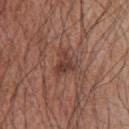Notes:
* TBP lesion metrics · a footprint of about 4.5 mm² and a shape eccentricity near 0.7; a mean CIELAB color near L≈39 a*≈21 b*≈25, a lesion–skin lightness drop of about 9, and a normalized border contrast of about 7.5; a border-irregularity rating of about 6/10 and internal color variation of about 2 on a 0–10 scale; a detector confidence of about 100 out of 100 that the crop contains a lesion
* lesion diameter · about 3 mm
* body site · the upper back
* imaging modality · ~15 mm tile from a whole-body skin photo
* patient · male, aged 63 to 67